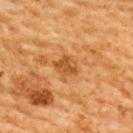This lesion was catalogued during total-body skin photography and was not selected for biopsy. Imaged with cross-polarized lighting. From the upper back. An algorithmic analysis of the crop reported an average lesion color of about L≈43 a*≈23 b*≈39 (CIELAB). And it measured a classifier nevus-likeness of about 0/100 and lesion-presence confidence of about 100/100. Measured at roughly 3 mm in maximum diameter. This image is a 15 mm lesion crop taken from a total-body photograph. A male patient, roughly 60 years of age.